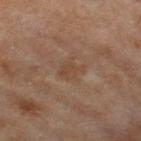Captured during whole-body skin photography for melanoma surveillance; the lesion was not biopsied.
A female patient, aged approximately 60.
Automated image analysis of the tile measured a lesion area of about 5 mm² and a shape-asymmetry score of about 0.3 (0 = symmetric). It also reported a mean CIELAB color near L≈40 a*≈16 b*≈27, roughly 5 lightness units darker than nearby skin, and a normalized border contrast of about 5. It also reported a color-variation rating of about 1.5/10 and a peripheral color-asymmetry measure near 0.5.
Longest diameter approximately 3 mm.
Imaged with cross-polarized lighting.
The lesion is on the leg.
This image is a 15 mm lesion crop taken from a total-body photograph.